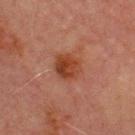Image and clinical context: Cropped from a total-body skin-imaging series; the visible field is about 15 mm. The total-body-photography lesion software estimated a footprint of about 8 mm², a shape eccentricity near 0.3, and two-axis asymmetry of about 0.15. And it measured a border-irregularity index near 1.5/10, a within-lesion color-variation index near 4.5/10, and radial color variation of about 1.5. And it measured a nevus-likeness score of about 80/100 and a lesion-detection confidence of about 100/100. Captured under cross-polarized illumination. A male patient in their 70s. The lesion is on the chest. The lesion's longest dimension is about 3 mm.